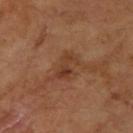Q: Was this lesion biopsied?
A: total-body-photography surveillance lesion; no biopsy
Q: What is the lesion's diameter?
A: about 3.5 mm
Q: How was this image acquired?
A: ~15 mm tile from a whole-body skin photo
Q: Who is the patient?
A: female, about 70 years old
Q: How was the tile lit?
A: cross-polarized illumination
Q: Lesion location?
A: the left forearm